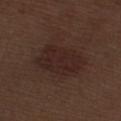This lesion was catalogued during total-body skin photography and was not selected for biopsy. Located on the right thigh. A region of skin cropped from a whole-body photographic capture, roughly 15 mm wide. A male subject, aged around 70. Approximately 5.5 mm at its widest.